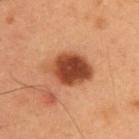Impression:
Part of a total-body skin-imaging series; this lesion was reviewed on a skin check and was not flagged for biopsy.
Acquisition and patient details:
Captured under cross-polarized illumination. A 15 mm crop from a total-body photograph taken for skin-cancer surveillance. A male patient, in their mid- to late 50s. From the upper back.